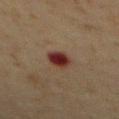  biopsy_status: not biopsied; imaged during a skin examination
  automated_metrics:
    border_irregularity_0_10: 2.0
    color_variation_0_10: 4.0
    nevus_likeness_0_100: 0
    lesion_detection_confidence_0_100: 100
  patient:
    sex: male
    age_approx: 60
  image:
    source: total-body photography crop
    field_of_view_mm: 15
  site: chest
  lighting: cross-polarized
  lesion_size:
    long_diameter_mm_approx: 3.0